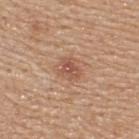Q: Was this lesion biopsied?
A: no biopsy performed (imaged during a skin exam)
Q: How large is the lesion?
A: about 3 mm
Q: Automated lesion metrics?
A: a nevus-likeness score of about 40/100
Q: Lesion location?
A: the upper back
Q: How was this image acquired?
A: total-body-photography crop, ~15 mm field of view
Q: Illumination type?
A: white-light illumination
Q: What are the patient's age and sex?
A: male, aged approximately 55